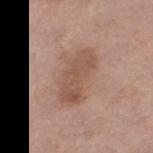This lesion was catalogued during total-body skin photography and was not selected for biopsy. Located on the left leg. About 6 mm across. Captured under white-light illumination. A region of skin cropped from a whole-body photographic capture, roughly 15 mm wide. The subject is a female in their mid-60s.